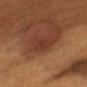The lesion was photographed on a routine skin check and not biopsied; there is no pathology result.
The lesion is located on the left upper arm.
The total-body-photography lesion software estimated an average lesion color of about L≈28 a*≈19 b*≈24 (CIELAB) and a lesion–skin lightness drop of about 5.
Measured at roughly 3.5 mm in maximum diameter.
A close-up tile cropped from a whole-body skin photograph, about 15 mm across.
A female patient, approximately 55 years of age.